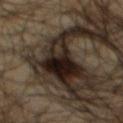workup — no biopsy performed (imaged during a skin exam) | size — ≈9 mm | lighting — cross-polarized | TBP lesion metrics — a normalized lesion–skin contrast near 18.5; border irregularity of about 6.5 on a 0–10 scale, internal color variation of about 9.5 on a 0–10 scale, and radial color variation of about 3.5; a classifier nevus-likeness of about 80/100 and lesion-presence confidence of about 5/100 | patient — male, approximately 65 years of age | imaging modality — total-body-photography crop, ~15 mm field of view | anatomic site — the mid back.